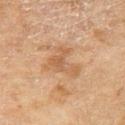| field | value |
|---|---|
| follow-up | total-body-photography surveillance lesion; no biopsy |
| tile lighting | cross-polarized |
| imaging modality | ~15 mm crop, total-body skin-cancer survey |
| site | the right thigh |
| patient | female, about 60 years old |
| lesion size | ~4.5 mm (longest diameter) |